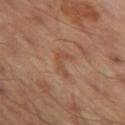follow-up: no biopsy performed (imaged during a skin exam); patient: male, aged around 45; site: the left thigh; imaging modality: ~15 mm crop, total-body skin-cancer survey; lesion diameter: ~3 mm (longest diameter); illumination: cross-polarized.